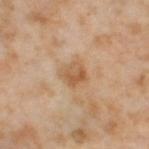{
  "biopsy_status": "not biopsied; imaged during a skin examination",
  "patient": {
    "sex": "female",
    "age_approx": 55
  },
  "lesion_size": {
    "long_diameter_mm_approx": 3.0
  },
  "site": "leg",
  "lighting": "cross-polarized",
  "automated_metrics": {
    "cielab_L": 59,
    "cielab_a": 19,
    "cielab_b": 36,
    "vs_skin_contrast_norm": 6.5
  },
  "image": {
    "source": "total-body photography crop",
    "field_of_view_mm": 15
  }
}